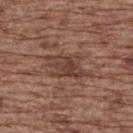Impression: Part of a total-body skin-imaging series; this lesion was reviewed on a skin check and was not flagged for biopsy. Background: A female subject, roughly 75 years of age. The total-body-photography lesion software estimated a shape eccentricity near 0.85 and a symmetry-axis asymmetry near 0.35. And it measured a mean CIELAB color near L≈41 a*≈20 b*≈24 and a lesion-to-skin contrast of about 7 (normalized; higher = more distinct). The analysis additionally found border irregularity of about 5 on a 0–10 scale and internal color variation of about 3.5 on a 0–10 scale. A 15 mm close-up extracted from a 3D total-body photography capture. From the upper back.